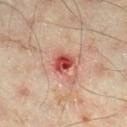<case>
  <biopsy_status>not biopsied; imaged during a skin examination</biopsy_status>
  <automated_metrics>
    <area_mm2_approx>5.0</area_mm2_approx>
    <eccentricity>0.7</eccentricity>
    <shape_asymmetry>0.25</shape_asymmetry>
    <border_irregularity_0_10>2.5</border_irregularity_0_10>
    <color_variation_0_10>6.0</color_variation_0_10>
    <nevus_likeness_0_100>0</nevus_likeness_0_100>
    <lesion_detection_confidence_0_100>100</lesion_detection_confidence_0_100>
  </automated_metrics>
  <image>
    <source>total-body photography crop</source>
    <field_of_view_mm>15</field_of_view_mm>
  </image>
  <site>right lower leg</site>
  <lighting>cross-polarized</lighting>
  <patient>
    <sex>male</sex>
    <age_approx>45</age_approx>
  </patient>
  <lesion_size>
    <long_diameter_mm_approx>3.0</long_diameter_mm_approx>
  </lesion_size>
</case>